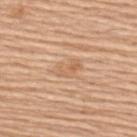Notes:
• follow-up · catalogued during a skin exam; not biopsied
• illumination · white-light illumination
• imaging modality · ~15 mm crop, total-body skin-cancer survey
• body site · the upper back
• subject · female, about 60 years old
• lesion diameter · ~3.5 mm (longest diameter)